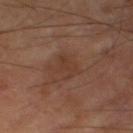{"biopsy_status": "not biopsied; imaged during a skin examination", "image": {"source": "total-body photography crop", "field_of_view_mm": 15}, "patient": {"sex": "male", "age_approx": 65}, "lighting": "cross-polarized", "automated_metrics": {"eccentricity": 0.45, "shape_asymmetry": 0.3, "border_irregularity_0_10": 3.5, "peripheral_color_asymmetry": 0.5, "nevus_likeness_0_100": 0, "lesion_detection_confidence_0_100": 100}, "lesion_size": {"long_diameter_mm_approx": 3.0}, "site": "right thigh"}